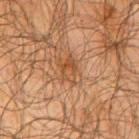Impression: No biopsy was performed on this lesion — it was imaged during a full skin examination and was not determined to be concerning. Acquisition and patient details: On the arm. Imaged with cross-polarized lighting. A male subject approximately 65 years of age. Measured at roughly 3 mm in maximum diameter. Cropped from a whole-body photographic skin survey; the tile spans about 15 mm.